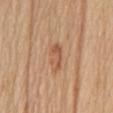<record>
  <biopsy_status>not biopsied; imaged during a skin examination</biopsy_status>
  <patient>
    <sex>female</sex>
    <age_approx>70</age_approx>
  </patient>
  <site>back</site>
  <image>
    <source>total-body photography crop</source>
    <field_of_view_mm>15</field_of_view_mm>
  </image>
  <automated_metrics>
    <color_variation_0_10>1.0</color_variation_0_10>
    <peripheral_color_asymmetry>0.5</peripheral_color_asymmetry>
  </automated_metrics>
  <lesion_size>
    <long_diameter_mm_approx>3.5</long_diameter_mm_approx>
  </lesion_size>
</record>